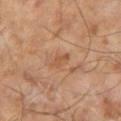subject = male, aged 63 to 67; lesion size = ~2.5 mm (longest diameter); image = ~15 mm crop, total-body skin-cancer survey.